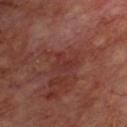No biopsy was performed on this lesion — it was imaged during a full skin examination and was not determined to be concerning. The lesion is located on the chest. A male patient, aged 68 to 72. Cropped from a total-body skin-imaging series; the visible field is about 15 mm.